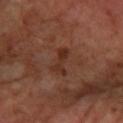Part of a total-body skin-imaging series; this lesion was reviewed on a skin check and was not flagged for biopsy.
Located on the right forearm.
Captured under cross-polarized illumination.
The recorded lesion diameter is about 3.5 mm.
A female patient aged 63–67.
A lesion tile, about 15 mm wide, cut from a 3D total-body photograph.